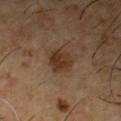biopsy status = imaged on a skin check; not biopsied
illumination = cross-polarized
body site = the front of the torso
automated lesion analysis = roughly 8 lightness units darker than nearby skin and a normalized border contrast of about 8.5
subject = male, about 55 years old
lesion size = ≈3 mm
imaging modality = ~15 mm crop, total-body skin-cancer survey